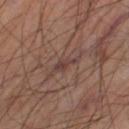This lesion was catalogued during total-body skin photography and was not selected for biopsy. Cropped from a whole-body photographic skin survey; the tile spans about 15 mm. A male patient, aged 63 to 67. Approximately 2.5 mm at its widest. Automated tile analysis of the lesion measured a classifier nevus-likeness of about 0/100 and a lesion-detection confidence of about 70/100. The tile uses cross-polarized illumination. The lesion is on the left thigh.